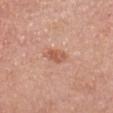biopsy status=imaged on a skin check; not biopsied